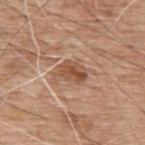Impression:
Part of a total-body skin-imaging series; this lesion was reviewed on a skin check and was not flagged for biopsy.
Clinical summary:
A male patient aged 58–62. The lesion is on the upper back. A lesion tile, about 15 mm wide, cut from a 3D total-body photograph. The total-body-photography lesion software estimated a lesion area of about 6 mm², an eccentricity of roughly 0.75, and two-axis asymmetry of about 0.3. The analysis additionally found a peripheral color-asymmetry measure near 1.5. The software also gave an automated nevus-likeness rating near 10 out of 100 and lesion-presence confidence of about 100/100.